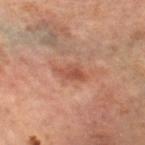Recorded during total-body skin imaging; not selected for excision or biopsy. A 15 mm crop from a total-body photograph taken for skin-cancer surveillance. The subject is a female in their mid- to late 60s. The lesion's longest dimension is about 3.5 mm. From the leg. Automated image analysis of the tile measured a lesion area of about 4.5 mm², an eccentricity of roughly 0.85, and a symmetry-axis asymmetry near 0.3. And it measured a normalized border contrast of about 7.